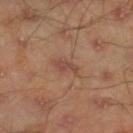Impression: Part of a total-body skin-imaging series; this lesion was reviewed on a skin check and was not flagged for biopsy. Context: The total-body-photography lesion software estimated a mean CIELAB color near L≈45 a*≈20 b*≈27, roughly 7 lightness units darker than nearby skin, and a normalized border contrast of about 6. And it measured border irregularity of about 4.5 on a 0–10 scale, internal color variation of about 0.5 on a 0–10 scale, and radial color variation of about 0. And it measured an automated nevus-likeness rating near 20 out of 100. On the right lower leg. Cropped from a total-body skin-imaging series; the visible field is about 15 mm. This is a cross-polarized tile. A male subject approximately 45 years of age. Longest diameter approximately 2.5 mm.